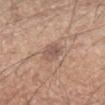Q: Is there a histopathology result?
A: imaged on a skin check; not biopsied
Q: What kind of image is this?
A: 15 mm crop, total-body photography
Q: Illumination type?
A: white-light illumination
Q: What is the lesion's diameter?
A: ~2.5 mm (longest diameter)
Q: Who is the patient?
A: male, aged 58 to 62
Q: Lesion location?
A: the left forearm